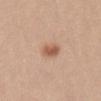This lesion was catalogued during total-body skin photography and was not selected for biopsy. The lesion is located on the abdomen. A female subject in their mid- to late 20s. Captured under white-light illumination. An algorithmic analysis of the crop reported a lesion color around L≈58 a*≈22 b*≈31 in CIELAB, about 11 CIELAB-L* units darker than the surrounding skin, and a lesion-to-skin contrast of about 7.5 (normalized; higher = more distinct). It also reported an automated nevus-likeness rating near 100 out of 100 and lesion-presence confidence of about 100/100. A close-up tile cropped from a whole-body skin photograph, about 15 mm across.